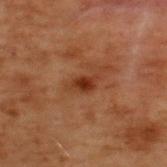notes — total-body-photography surveillance lesion; no biopsy
acquisition — 15 mm crop, total-body photography
site — the back
tile lighting — cross-polarized
lesion diameter — about 2.5 mm
subject — male, aged approximately 70
image-analysis metrics — an outline eccentricity of about 0.8 (0 = round, 1 = elongated) and a shape-asymmetry score of about 0.3 (0 = symmetric)